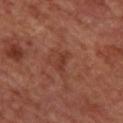Imaged during a routine full-body skin examination; the lesion was not biopsied and no histopathology is available.
Measured at roughly 3 mm in maximum diameter.
On the back.
Captured under cross-polarized illumination.
A roughly 15 mm field-of-view crop from a total-body skin photograph.
Automated tile analysis of the lesion measured a footprint of about 2.5 mm² and a shape eccentricity near 0.9. And it measured a mean CIELAB color near L≈35 a*≈25 b*≈28, roughly 6 lightness units darker than nearby skin, and a lesion-to-skin contrast of about 6 (normalized; higher = more distinct). The analysis additionally found a within-lesion color-variation index near 0/10 and a peripheral color-asymmetry measure near 0.
The patient is a female aged 43–47.